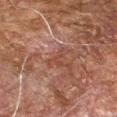No biopsy was performed on this lesion — it was imaged during a full skin examination and was not determined to be concerning. This image is a 15 mm lesion crop taken from a total-body photograph. Longest diameter approximately 2.5 mm. Imaged with cross-polarized lighting. The lesion is on the right forearm. A male patient, aged around 70.